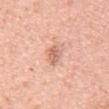Q: Is there a histopathology result?
A: total-body-photography surveillance lesion; no biopsy
Q: How large is the lesion?
A: ≈3 mm
Q: Patient demographics?
A: male, approximately 55 years of age
Q: How was the tile lit?
A: white-light illumination
Q: What kind of image is this?
A: 15 mm crop, total-body photography
Q: What is the anatomic site?
A: the abdomen